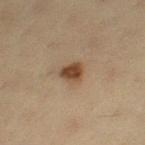| key | value |
|---|---|
| notes | catalogued during a skin exam; not biopsied |
| imaging modality | ~15 mm crop, total-body skin-cancer survey |
| patient | female, about 40 years old |
| illumination | cross-polarized illumination |
| body site | the left thigh |